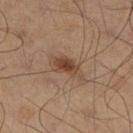| field | value |
|---|---|
| notes | no biopsy performed (imaged during a skin exam) |
| acquisition | total-body-photography crop, ~15 mm field of view |
| patient | male, about 50 years old |
| location | the left leg |